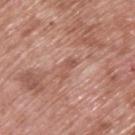Part of a total-body skin-imaging series; this lesion was reviewed on a skin check and was not flagged for biopsy. The recorded lesion diameter is about 3 mm. A male subject, approximately 70 years of age. Imaged with white-light lighting. The lesion is located on the back. A 15 mm close-up tile from a total-body photography series done for melanoma screening.